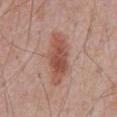Q: Is there a histopathology result?
A: catalogued during a skin exam; not biopsied
Q: What is the anatomic site?
A: the abdomen
Q: What is the imaging modality?
A: ~15 mm tile from a whole-body skin photo
Q: What are the patient's age and sex?
A: male, approximately 70 years of age